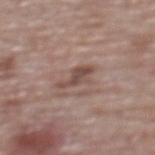Case summary:
– follow-up: imaged on a skin check; not biopsied
– automated metrics: an area of roughly 5 mm² and an eccentricity of roughly 0.85; an average lesion color of about L≈48 a*≈18 b*≈22 (CIELAB) and a lesion-to-skin contrast of about 7.5 (normalized; higher = more distinct)
– acquisition: total-body-photography crop, ~15 mm field of view
– patient: female, in their mid-60s
– lesion diameter: about 3.5 mm
– lighting: white-light illumination
– location: the upper back